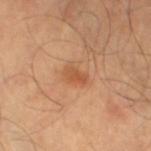Part of a total-body skin-imaging series; this lesion was reviewed on a skin check and was not flagged for biopsy. A roughly 15 mm field-of-view crop from a total-body skin photograph. The recorded lesion diameter is about 3 mm. The patient is a male roughly 45 years of age. The lesion-visualizer software estimated a lesion area of about 4 mm². It also reported border irregularity of about 2.5 on a 0–10 scale and internal color variation of about 2.5 on a 0–10 scale. The analysis additionally found an automated nevus-likeness rating near 70 out of 100 and lesion-presence confidence of about 100/100. This is a cross-polarized tile. Located on the left thigh.